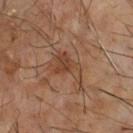Recorded during total-body skin imaging; not selected for excision or biopsy.
A male subject aged around 60.
A region of skin cropped from a whole-body photographic capture, roughly 15 mm wide.
This is a cross-polarized tile.
On the chest.